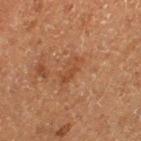workup — total-body-photography surveillance lesion; no biopsy
tile lighting — cross-polarized illumination
subject — male, aged approximately 75
body site — the left thigh
lesion diameter — ~3.5 mm (longest diameter)
acquisition — ~15 mm tile from a whole-body skin photo